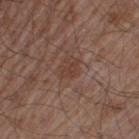{
  "biopsy_status": "not biopsied; imaged during a skin examination",
  "patient": {
    "sex": "male",
    "age_approx": 55
  },
  "lighting": "white-light",
  "automated_metrics": {
    "area_mm2_approx": 3.5,
    "eccentricity": 0.8,
    "shape_asymmetry": 0.3,
    "cielab_L": 39,
    "cielab_a": 19,
    "cielab_b": 25,
    "vs_skin_darker_L": 6.0,
    "vs_skin_contrast_norm": 5.5,
    "nevus_likeness_0_100": 0,
    "lesion_detection_confidence_0_100": 100
  },
  "image": {
    "source": "total-body photography crop",
    "field_of_view_mm": 15
  },
  "site": "left thigh"
}